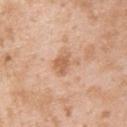Notes:
• workup · no biopsy performed (imaged during a skin exam)
• automated lesion analysis · an area of roughly 5.5 mm², an eccentricity of roughly 0.7, and a symmetry-axis asymmetry near 0.3; an average lesion color of about L≈62 a*≈21 b*≈34 (CIELAB), a lesion–skin lightness drop of about 10, and a normalized lesion–skin contrast near 6.5; a color-variation rating of about 2/10; an automated nevus-likeness rating near 0 out of 100 and a detector confidence of about 100 out of 100 that the crop contains a lesion
• acquisition · total-body-photography crop, ~15 mm field of view
• lighting · white-light
• subject · male, aged around 25
• location · the back